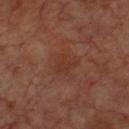notes: total-body-photography surveillance lesion; no biopsy | illumination: cross-polarized illumination | body site: the chest | subject: male, approximately 70 years of age | image source: ~15 mm tile from a whole-body skin photo | lesion diameter: about 3 mm.